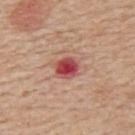Recorded during total-body skin imaging; not selected for excision or biopsy.
A 15 mm crop from a total-body photograph taken for skin-cancer surveillance.
A female subject, in their 50s.
This is a white-light tile.
Automated tile analysis of the lesion measured an average lesion color of about L≈49 a*≈35 b*≈25 (CIELAB), roughly 16 lightness units darker than nearby skin, and a normalized lesion–skin contrast near 11. And it measured a classifier nevus-likeness of about 0/100 and a lesion-detection confidence of about 100/100.
The recorded lesion diameter is about 3 mm.
Located on the upper back.